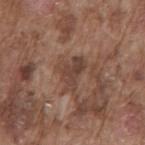| field | value |
|---|---|
| biopsy status | imaged on a skin check; not biopsied |
| imaging modality | 15 mm crop, total-body photography |
| lighting | white-light |
| location | the mid back |
| patient | male, in their mid-70s |